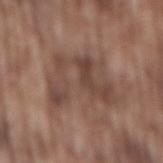Clinical impression:
Part of a total-body skin-imaging series; this lesion was reviewed on a skin check and was not flagged for biopsy.
Image and clinical context:
A close-up tile cropped from a whole-body skin photograph, about 15 mm across. On the mid back. Automated image analysis of the tile measured a lesion area of about 22 mm², a shape eccentricity near 0.75, and a shape-asymmetry score of about 0.5 (0 = symmetric). It also reported a mean CIELAB color near L≈44 a*≈17 b*≈24 and about 8 CIELAB-L* units darker than the surrounding skin. It also reported an automated nevus-likeness rating near 0 out of 100. A male patient, aged 73–77. Longest diameter approximately 7.5 mm. Imaged with white-light lighting.